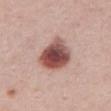{"biopsy_status": "not biopsied; imaged during a skin examination", "image": {"source": "total-body photography crop", "field_of_view_mm": 15}, "patient": {"sex": "female", "age_approx": 35}, "automated_metrics": {"area_mm2_approx": 14.0, "eccentricity": 0.55, "shape_asymmetry": 0.25, "cielab_L": 50, "cielab_a": 24, "cielab_b": 23, "vs_skin_darker_L": 19.0, "nevus_likeness_0_100": 95, "lesion_detection_confidence_0_100": 100}, "site": "upper back", "lesion_size": {"long_diameter_mm_approx": 4.5}, "lighting": "white-light"}